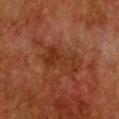Notes:
* notes — catalogued during a skin exam; not biopsied
* acquisition — ~15 mm tile from a whole-body skin photo
* patient — female, aged 48 to 52
* site — the chest